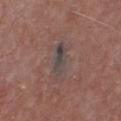| field | value |
|---|---|
| workup | catalogued during a skin exam; not biopsied |
| subject | male, aged approximately 55 |
| lesion size | about 4.5 mm |
| TBP lesion metrics | an area of roughly 6.5 mm², a shape eccentricity near 0.9, and a symmetry-axis asymmetry near 0.15 |
| location | the chest |
| acquisition | ~15 mm crop, total-body skin-cancer survey |